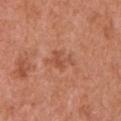Notes:
- workup: no biopsy performed (imaged during a skin exam)
- illumination: white-light illumination
- image: ~15 mm tile from a whole-body skin photo
- patient: male, roughly 65 years of age
- image-analysis metrics: two-axis asymmetry of about 0.4; a lesion color around L≈52 a*≈27 b*≈34 in CIELAB and about 7 CIELAB-L* units darker than the surrounding skin; an automated nevus-likeness rating near 0 out of 100 and a lesion-detection confidence of about 100/100
- lesion diameter: about 3.5 mm
- body site: the left upper arm